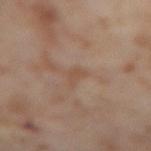notes=no biopsy performed (imaged during a skin exam)
image source=~15 mm crop, total-body skin-cancer survey
size=≈2.5 mm
subject=female, roughly 55 years of age
image-analysis metrics=a lesion area of about 2.5 mm², a shape eccentricity near 0.85, and two-axis asymmetry of about 0.6; a lesion color around L≈51 a*≈18 b*≈29 in CIELAB, about 5 CIELAB-L* units darker than the surrounding skin, and a normalized lesion–skin contrast near 5.5; a nevus-likeness score of about 0/100 and lesion-presence confidence of about 100/100
location=the leg